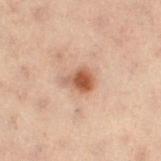Part of a total-body skin-imaging series; this lesion was reviewed on a skin check and was not flagged for biopsy. Located on the left leg. A female subject, aged around 55. Longest diameter approximately 3 mm. Cropped from a whole-body photographic skin survey; the tile spans about 15 mm. This is a cross-polarized tile.